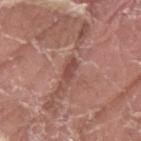Impression:
This lesion was catalogued during total-body skin photography and was not selected for biopsy.
Clinical summary:
About 3 mm across. On the left thigh. A 15 mm close-up extracted from a 3D total-body photography capture. Captured under white-light illumination. The subject is a male aged 38 to 42.